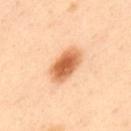Imaged during a routine full-body skin examination; the lesion was not biopsied and no histopathology is available.
The patient is a male roughly 45 years of age.
The lesion-visualizer software estimated a footprint of about 11 mm² and a shape-asymmetry score of about 0.15 (0 = symmetric). And it measured a mean CIELAB color near L≈64 a*≈26 b*≈41, a lesion–skin lightness drop of about 17, and a normalized border contrast of about 10. It also reported border irregularity of about 1.5 on a 0–10 scale and a peripheral color-asymmetry measure near 1.5. It also reported a classifier nevus-likeness of about 100/100.
From the upper back.
Imaged with cross-polarized lighting.
About 5 mm across.
This image is a 15 mm lesion crop taken from a total-body photograph.